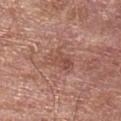The lesion is on the right upper arm. A male patient, in their mid- to late 70s. A 15 mm crop from a total-body photograph taken for skin-cancer surveillance. This is a white-light tile. Automated image analysis of the tile measured border irregularity of about 4.5 on a 0–10 scale, a within-lesion color-variation index near 4/10, and a peripheral color-asymmetry measure near 1.5.